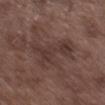Case summary:
- follow-up — total-body-photography surveillance lesion; no biopsy
- body site — the left thigh
- subject — male, about 75 years old
- imaging modality — ~15 mm crop, total-body skin-cancer survey
- illumination — white-light
- diameter — ≈5.5 mm